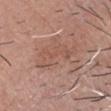Assessment: Captured during whole-body skin photography for melanoma surveillance; the lesion was not biopsied. Clinical summary: The subject is a male in their mid- to late 70s. On the head or neck. A roughly 15 mm field-of-view crop from a total-body skin photograph.